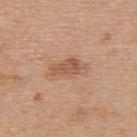Q: Was this lesion biopsied?
A: total-body-photography surveillance lesion; no biopsy
Q: What is the imaging modality?
A: ~15 mm crop, total-body skin-cancer survey
Q: Automated lesion metrics?
A: a footprint of about 7 mm² and a shape-asymmetry score of about 0.25 (0 = symmetric); a lesion color around L≈56 a*≈21 b*≈33 in CIELAB, a lesion–skin lightness drop of about 9, and a normalized lesion–skin contrast near 6.5
Q: Lesion size?
A: ≈4 mm
Q: Who is the patient?
A: male, in their 70s
Q: Where on the body is the lesion?
A: the upper back
Q: What lighting was used for the tile?
A: white-light